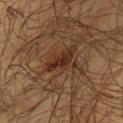Case summary:
- follow-up — no biopsy performed (imaged during a skin exam)
- imaging modality — 15 mm crop, total-body photography
- patient — male, aged 43–47
- lesion diameter — ~3.5 mm (longest diameter)
- anatomic site — the chest
- TBP lesion metrics — an eccentricity of roughly 0.95 and two-axis asymmetry of about 0.35
- lighting — cross-polarized